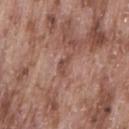| key | value |
|---|---|
| workup | total-body-photography surveillance lesion; no biopsy |
| site | the back |
| illumination | white-light |
| subject | male, about 75 years old |
| acquisition | total-body-photography crop, ~15 mm field of view |
| diameter | ~3 mm (longest diameter) |